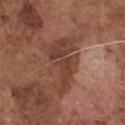Image and clinical context:
The total-body-photography lesion software estimated an eccentricity of roughly 0.9 and a shape-asymmetry score of about 0.5 (0 = symmetric). The analysis additionally found an average lesion color of about L≈41 a*≈22 b*≈27 (CIELAB), roughly 9 lightness units darker than nearby skin, and a lesion-to-skin contrast of about 7.5 (normalized; higher = more distinct). Cropped from a total-body skin-imaging series; the visible field is about 15 mm. The lesion is located on the chest. A male subject, aged 73–77. This is a white-light tile.